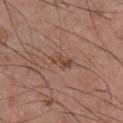<case>
<biopsy_status>not biopsied; imaged during a skin examination</biopsy_status>
<image>
  <source>total-body photography crop</source>
  <field_of_view_mm>15</field_of_view_mm>
</image>
<lighting>white-light</lighting>
<lesion_size>
  <long_diameter_mm_approx>3.0</long_diameter_mm_approx>
</lesion_size>
<site>chest</site>
<patient>
  <sex>male</sex>
  <age_approx>40</age_approx>
</patient>
<automated_metrics>
  <area_mm2_approx>3.5</area_mm2_approx>
  <shape_asymmetry>0.25</shape_asymmetry>
  <cielab_L>46</cielab_L>
  <cielab_a>21</cielab_a>
  <cielab_b>27</cielab_b>
  <vs_skin_darker_L>8.0</vs_skin_darker_L>
  <vs_skin_contrast_norm>6.5</vs_skin_contrast_norm>
  <lesion_detection_confidence_0_100>100</lesion_detection_confidence_0_100>
</automated_metrics>
</case>